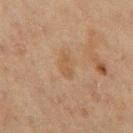Q: Is there a histopathology result?
A: no biopsy performed (imaged during a skin exam)
Q: What are the patient's age and sex?
A: female, in their 50s
Q: What is the imaging modality?
A: total-body-photography crop, ~15 mm field of view
Q: What is the anatomic site?
A: the left thigh